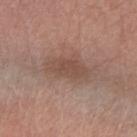Acquisition and patient details:
About 5.5 mm across. On the right forearm. A roughly 15 mm field-of-view crop from a total-body skin photograph. A female patient aged 63 to 67.